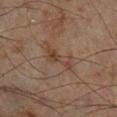Clinical impression: Imaged during a routine full-body skin examination; the lesion was not biopsied and no histopathology is available. Context: The lesion is on the left lower leg. A male patient aged around 65. A region of skin cropped from a whole-body photographic capture, roughly 15 mm wide. This is a cross-polarized tile.